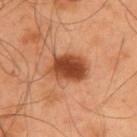Assessment: Recorded during total-body skin imaging; not selected for excision or biopsy. Clinical summary: A region of skin cropped from a whole-body photographic capture, roughly 15 mm wide. Located on the upper back. This is a cross-polarized tile. A male patient, aged 53 to 57. Longest diameter approximately 5 mm. Automated image analysis of the tile measured an area of roughly 13 mm², an eccentricity of roughly 0.75, and two-axis asymmetry of about 0.2. The analysis additionally found an average lesion color of about L≈46 a*≈27 b*≈36 (CIELAB) and about 16 CIELAB-L* units darker than the surrounding skin. The software also gave border irregularity of about 2 on a 0–10 scale and a peripheral color-asymmetry measure near 1.5. And it measured a detector confidence of about 100 out of 100 that the crop contains a lesion.